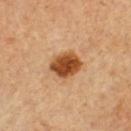Assessment:
The lesion was photographed on a routine skin check and not biopsied; there is no pathology result.
Image and clinical context:
From the front of the torso. A close-up tile cropped from a whole-body skin photograph, about 15 mm across. A male patient, aged approximately 65.